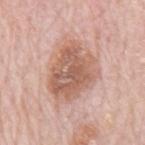| feature | finding |
|---|---|
| follow-up | imaged on a skin check; not biopsied |
| body site | the back |
| image | 15 mm crop, total-body photography |
| illumination | white-light |
| patient | male, approximately 80 years of age |